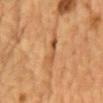<lesion>
  <biopsy_status>not biopsied; imaged during a skin examination</biopsy_status>
  <lighting>cross-polarized</lighting>
  <image>
    <source>total-body photography crop</source>
    <field_of_view_mm>15</field_of_view_mm>
  </image>
  <automated_metrics>
    <area_mm2_approx>6.0</area_mm2_approx>
    <eccentricity>0.95</eccentricity>
    <shape_asymmetry>0.3</shape_asymmetry>
    <cielab_L>48</cielab_L>
    <cielab_a>20</cielab_a>
    <cielab_b>35</cielab_b>
    <vs_skin_contrast_norm>6.0</vs_skin_contrast_norm>
    <border_irregularity_0_10>5.0</border_irregularity_0_10>
    <color_variation_0_10>7.5</color_variation_0_10>
    <peripheral_color_asymmetry>3.0</peripheral_color_asymmetry>
  </automated_metrics>
  <lesion_size>
    <long_diameter_mm_approx>5.0</long_diameter_mm_approx>
  </lesion_size>
  <site>chest</site>
  <patient>
    <sex>male</sex>
    <age_approx>85+</age_approx>
  </patient>
</lesion>